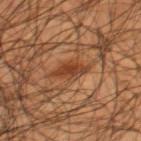From the right thigh. This is a cross-polarized tile. A lesion tile, about 15 mm wide, cut from a 3D total-body photograph. The recorded lesion diameter is about 4 mm. Automated tile analysis of the lesion measured border irregularity of about 3 on a 0–10 scale, internal color variation of about 3.5 on a 0–10 scale, and peripheral color asymmetry of about 1. It also reported a lesion-detection confidence of about 80/100. A male patient, aged 43–47.